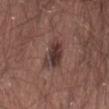biopsy_status: not biopsied; imaged during a skin examination
lesion_size:
  long_diameter_mm_approx: 4.5
lighting: white-light
patient:
  sex: male
  age_approx: 25
site: lower back
image:
  source: total-body photography crop
  field_of_view_mm: 15
automated_metrics:
  area_mm2_approx: 8.5
  eccentricity: 0.85
  shape_asymmetry: 0.3
  cielab_L: 36
  cielab_a: 17
  cielab_b: 19
  vs_skin_darker_L: 10.0
  vs_skin_contrast_norm: 9.5
  nevus_likeness_0_100: 50